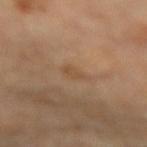biopsy_status: not biopsied; imaged during a skin examination
lesion_size:
  long_diameter_mm_approx: 2.5
lighting: cross-polarized
patient:
  sex: female
  age_approx: 65
image:
  source: total-body photography crop
  field_of_view_mm: 15
site: arm